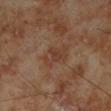A male subject, roughly 70 years of age. The lesion is located on the left lower leg. A 15 mm close-up tile from a total-body photography series done for melanoma screening. Measured at roughly 4 mm in maximum diameter. Captured under cross-polarized illumination.